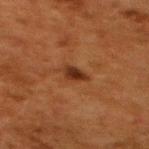Assessment:
Recorded during total-body skin imaging; not selected for excision or biopsy.
Context:
Cropped from a total-body skin-imaging series; the visible field is about 15 mm. A female patient, in their 50s. The lesion is located on the upper back.